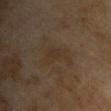Imaged during a routine full-body skin examination; the lesion was not biopsied and no histopathology is available.